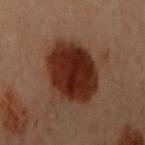<lesion>
  <lighting>cross-polarized</lighting>
  <site>left arm</site>
  <patient>
    <sex>female</sex>
    <age_approx>60</age_approx>
  </patient>
  <image>
    <source>total-body photography crop</source>
    <field_of_view_mm>15</field_of_view_mm>
  </image>
  <automated_metrics>
    <cielab_L>24</cielab_L>
    <cielab_a>21</cielab_a>
    <cielab_b>25</cielab_b>
    <vs_skin_darker_L>14.0</vs_skin_darker_L>
    <vs_skin_contrast_norm>14.0</vs_skin_contrast_norm>
    <border_irregularity_0_10>1.5</border_irregularity_0_10>
    <color_variation_0_10>4.0</color_variation_0_10>
    <peripheral_color_asymmetry>1.5</peripheral_color_asymmetry>
    <nevus_likeness_0_100>100</nevus_likeness_0_100>
  </automated_metrics>
  <lesion_size>
    <long_diameter_mm_approx>7.0</long_diameter_mm_approx>
  </lesion_size>
</lesion>